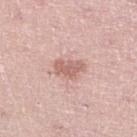| feature | finding |
|---|---|
| notes | no biopsy performed (imaged during a skin exam) |
| subject | female, aged around 40 |
| image-analysis metrics | an area of roughly 6 mm², an outline eccentricity of about 0.75 (0 = round, 1 = elongated), and a symmetry-axis asymmetry near 0.35; a normalized lesion–skin contrast near 7 |
| tile lighting | white-light illumination |
| imaging modality | ~15 mm crop, total-body skin-cancer survey |
| anatomic site | the right lower leg |